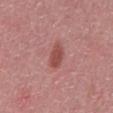<lesion>
  <biopsy_status>not biopsied; imaged during a skin examination</biopsy_status>
</lesion>